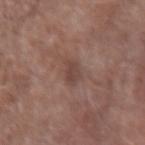Q: Was this lesion biopsied?
A: imaged on a skin check; not biopsied
Q: Lesion location?
A: the right forearm
Q: Who is the patient?
A: male, aged 68–72
Q: Automated lesion metrics?
A: a lesion area of about 4 mm², an eccentricity of roughly 0.8, and a shape-asymmetry score of about 0.3 (0 = symmetric); an average lesion color of about L≈43 a*≈18 b*≈22 (CIELAB), roughly 8 lightness units darker than nearby skin, and a normalized lesion–skin contrast near 6.5; a within-lesion color-variation index near 1.5/10 and a peripheral color-asymmetry measure near 0.5
Q: What is the imaging modality?
A: total-body-photography crop, ~15 mm field of view